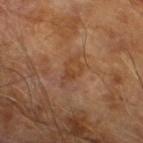workup: imaged on a skin check; not biopsied
acquisition: 15 mm crop, total-body photography
image-analysis metrics: an area of roughly 5.5 mm² and an outline eccentricity of about 0.85 (0 = round, 1 = elongated); a border-irregularity index near 3.5/10 and a within-lesion color-variation index near 3/10
patient: male, aged 63–67
illumination: cross-polarized